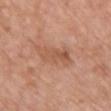biopsy status=imaged on a skin check; not biopsied
size=≈5 mm
body site=the chest
tile lighting=white-light illumination
image=15 mm crop, total-body photography
subject=female, aged approximately 65